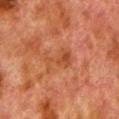follow-up: imaged on a skin check; not biopsied
lesion diameter: about 4 mm
acquisition: 15 mm crop, total-body photography
anatomic site: the left lower leg
patient: male, roughly 80 years of age